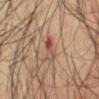Clinical summary: A male subject aged around 65. On the right forearm. A lesion tile, about 15 mm wide, cut from a 3D total-body photograph. Measured at roughly 3.5 mm in maximum diameter. This is a cross-polarized tile. The lesion-visualizer software estimated a lesion area of about 4 mm², a shape eccentricity near 0.9, and two-axis asymmetry of about 0.35. The software also gave a mean CIELAB color near L≈40 a*≈18 b*≈22, roughly 8 lightness units darker than nearby skin, and a normalized lesion–skin contrast near 6.5. It also reported a border-irregularity index near 4/10, internal color variation of about 6.5 on a 0–10 scale, and a peripheral color-asymmetry measure near 1.5. The software also gave an automated nevus-likeness rating near 0 out of 100 and a detector confidence of about 100 out of 100 that the crop contains a lesion.